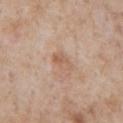No biopsy was performed on this lesion — it was imaged during a full skin examination and was not determined to be concerning. The lesion is located on the chest. A male subject in their mid- to late 60s. Automated tile analysis of the lesion measured a lesion area of about 3.5 mm², an eccentricity of roughly 0.85, and a shape-asymmetry score of about 0.3 (0 = symmetric). The analysis additionally found an average lesion color of about L≈59 a*≈19 b*≈31 (CIELAB), roughly 7 lightness units darker than nearby skin, and a normalized lesion–skin contrast near 6. And it measured a border-irregularity index near 3/10 and radial color variation of about 0.5. The software also gave a classifier nevus-likeness of about 0/100. Measured at roughly 3 mm in maximum diameter. The tile uses white-light illumination. A 15 mm close-up extracted from a 3D total-body photography capture.